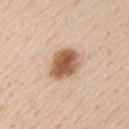follow-up = imaged on a skin check; not biopsied
anatomic site = the chest
imaging modality = total-body-photography crop, ~15 mm field of view
subject = male, aged around 60